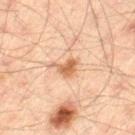biopsy status=total-body-photography surveillance lesion; no biopsy | automated metrics=a nevus-likeness score of about 80/100 and lesion-presence confidence of about 100/100 | image source=~15 mm tile from a whole-body skin photo | diameter=~3 mm (longest diameter) | subject=male, in their mid-40s | lighting=cross-polarized illumination | site=the leg.